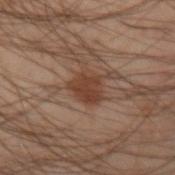biopsy status = total-body-photography surveillance lesion; no biopsy | size = ≈3.5 mm | patient = male, roughly 50 years of age | illumination = cross-polarized | image source = total-body-photography crop, ~15 mm field of view | automated metrics = a footprint of about 7.5 mm², an eccentricity of roughly 0.2, and two-axis asymmetry of about 0.25; a lesion-detection confidence of about 100/100 | anatomic site = the left arm.